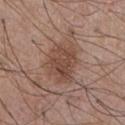The lesion was tiled from a total-body skin photograph and was not biopsied. This image is a 15 mm lesion crop taken from a total-body photograph. The tile uses white-light illumination. A male patient in their mid-50s. The lesion is on the chest. Measured at roughly 5 mm in maximum diameter. The total-body-photography lesion software estimated a border-irregularity rating of about 2.5/10 and a color-variation rating of about 3.5/10. The analysis additionally found a classifier nevus-likeness of about 30/100.